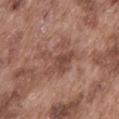Imaged during a routine full-body skin examination; the lesion was not biopsied and no histopathology is available.
This is a white-light tile.
The patient is a male aged around 75.
An algorithmic analysis of the crop reported an outline eccentricity of about 0.65 (0 = round, 1 = elongated) and a symmetry-axis asymmetry near 0.65. And it measured roughly 8 lightness units darker than nearby skin and a lesion-to-skin contrast of about 6 (normalized; higher = more distinct). The analysis additionally found an automated nevus-likeness rating near 0 out of 100 and a lesion-detection confidence of about 100/100.
The recorded lesion diameter is about 5 mm.
On the abdomen.
A 15 mm crop from a total-body photograph taken for skin-cancer surveillance.